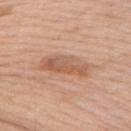* workup — imaged on a skin check; not biopsied
* size — about 6 mm
* automated lesion analysis — a mean CIELAB color near L≈58 a*≈22 b*≈32 and a normalized border contrast of about 6.5; a nevus-likeness score of about 5/100 and a detector confidence of about 100 out of 100 that the crop contains a lesion
* tile lighting — white-light
* image source — 15 mm crop, total-body photography
* subject — male, aged 78 to 82
* body site — the right upper arm